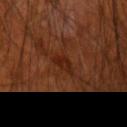Impression: Recorded during total-body skin imaging; not selected for excision or biopsy. Background: A male patient in their 70s. Longest diameter approximately 2.5 mm. This is a cross-polarized tile. A 15 mm close-up extracted from a 3D total-body photography capture. An algorithmic analysis of the crop reported an outline eccentricity of about 0.75 (0 = round, 1 = elongated) and a shape-asymmetry score of about 0.35 (0 = symmetric). The software also gave a border-irregularity rating of about 3/10 and a peripheral color-asymmetry measure near 0.5. From the right upper arm.